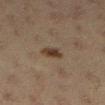Q: Was this lesion biopsied?
A: catalogued during a skin exam; not biopsied
Q: Who is the patient?
A: female, approximately 20 years of age
Q: How large is the lesion?
A: ~2.5 mm (longest diameter)
Q: What did automated image analysis measure?
A: a footprint of about 4 mm², a shape eccentricity near 0.75, and a shape-asymmetry score of about 0.2 (0 = symmetric); a lesion color around L≈32 a*≈14 b*≈24 in CIELAB and about 10 CIELAB-L* units darker than the surrounding skin; a border-irregularity rating of about 2/10, internal color variation of about 3 on a 0–10 scale, and a peripheral color-asymmetry measure near 1; a classifier nevus-likeness of about 100/100
Q: Illumination type?
A: cross-polarized illumination
Q: How was this image acquired?
A: ~15 mm tile from a whole-body skin photo
Q: Lesion location?
A: the right lower leg